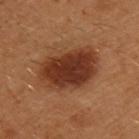workup: imaged on a skin check; not biopsied | size: about 6.5 mm | automated metrics: a lesion area of about 23 mm², a shape eccentricity near 0.75, and two-axis asymmetry of about 0.2 | body site: the upper back | illumination: cross-polarized | imaging modality: total-body-photography crop, ~15 mm field of view | patient: male, in their 30s.